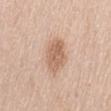Case summary:
- workup · total-body-photography surveillance lesion; no biopsy
- anatomic site · the left thigh
- acquisition · ~15 mm crop, total-body skin-cancer survey
- illumination · white-light
- lesion diameter · ≈4.5 mm
- patient · female, aged approximately 65